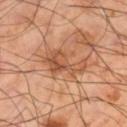Captured during whole-body skin photography for melanoma surveillance; the lesion was not biopsied. The patient is a male aged 48 to 52. The lesion's longest dimension is about 5.5 mm. A region of skin cropped from a whole-body photographic capture, roughly 15 mm wide. Located on the right lower leg.